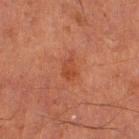{
  "biopsy_status": "not biopsied; imaged during a skin examination",
  "site": "left thigh",
  "patient": {
    "sex": "male",
    "age_approx": 65
  },
  "image": {
    "source": "total-body photography crop",
    "field_of_view_mm": 15
  }
}